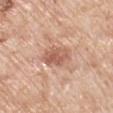{"biopsy_status": "not biopsied; imaged during a skin examination", "site": "left upper arm", "image": {"source": "total-body photography crop", "field_of_view_mm": 15}, "patient": {"sex": "male", "age_approx": 70}, "lesion_size": {"long_diameter_mm_approx": 4.0}, "lighting": "white-light"}